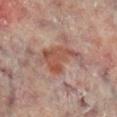Captured during whole-body skin photography for melanoma surveillance; the lesion was not biopsied. Imaged with cross-polarized lighting. A 15 mm crop from a total-body photograph taken for skin-cancer surveillance. About 6 mm across. The lesion is located on the leg. The lesion-visualizer software estimated an area of roughly 12 mm² and an eccentricity of roughly 0.8. And it measured an average lesion color of about L≈49 a*≈22 b*≈26 (CIELAB) and about 9 CIELAB-L* units darker than the surrounding skin. It also reported an automated nevus-likeness rating near 5 out of 100. A male patient, about 65 years old.